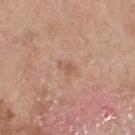The lesion's longest dimension is about 2 mm.
The lesion is on the upper back.
A 15 mm crop from a total-body photograph taken for skin-cancer surveillance.
Imaged with white-light lighting.
A male patient, aged approximately 55.